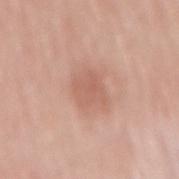Imaged during a routine full-body skin examination; the lesion was not biopsied and no histopathology is available. This is a white-light tile. Located on the abdomen. About 3.5 mm across. Cropped from a whole-body photographic skin survey; the tile spans about 15 mm. A female patient aged around 65.